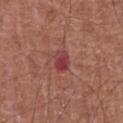Clinical impression: Part of a total-body skin-imaging series; this lesion was reviewed on a skin check and was not flagged for biopsy. Image and clinical context: Approximately 2.5 mm at its widest. The total-body-photography lesion software estimated roughly 9 lightness units darker than nearby skin and a normalized border contrast of about 8. A lesion tile, about 15 mm wide, cut from a 3D total-body photograph. The subject is a male aged around 65. Located on the chest. This is a white-light tile.